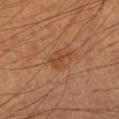follow-up: no biopsy performed (imaged during a skin exam).